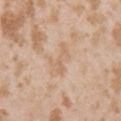{"biopsy_status": "not biopsied; imaged during a skin examination", "site": "left upper arm", "image": {"source": "total-body photography crop", "field_of_view_mm": 15}, "automated_metrics": {"vs_skin_darker_L": 6.0, "vs_skin_contrast_norm": 5.0}, "patient": {"sex": "female", "age_approx": 25}}